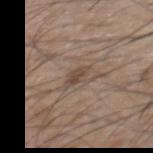{"biopsy_status": "not biopsied; imaged during a skin examination", "lesion_size": {"long_diameter_mm_approx": 2.5}, "site": "upper back", "patient": {"sex": "male", "age_approx": 55}, "image": {"source": "total-body photography crop", "field_of_view_mm": 15}}